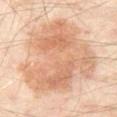Clinical impression: Imaged during a routine full-body skin examination; the lesion was not biopsied and no histopathology is available. Image and clinical context: A 15 mm close-up extracted from a 3D total-body photography capture. Located on the leg. A patient aged 53–57. About 10 mm across. An algorithmic analysis of the crop reported an area of roughly 65 mm², an outline eccentricity of about 0.4 (0 = round, 1 = elongated), and a shape-asymmetry score of about 0.3 (0 = symmetric). The analysis additionally found a mean CIELAB color near L≈68 a*≈20 b*≈34 and about 10 CIELAB-L* units darker than the surrounding skin. The analysis additionally found a border-irregularity rating of about 5/10, a color-variation rating of about 5/10, and a peripheral color-asymmetry measure near 1.5. Captured under cross-polarized illumination.